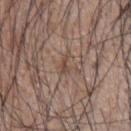{"biopsy_status": "not biopsied; imaged during a skin examination", "patient": {"sex": "male", "age_approx": 35}, "image": {"source": "total-body photography crop", "field_of_view_mm": 15}, "lighting": "white-light", "lesion_size": {"long_diameter_mm_approx": 2.5}, "site": "upper back"}